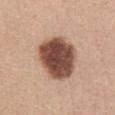<lesion>
  <biopsy_status>not biopsied; imaged during a skin examination</biopsy_status>
  <site>chest</site>
  <patient>
    <sex>female</sex>
    <age_approx>45</age_approx>
  </patient>
  <image>
    <source>total-body photography crop</source>
    <field_of_view_mm>15</field_of_view_mm>
  </image>
</lesion>